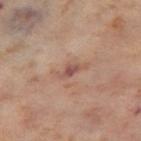Findings:
* notes · no biopsy performed (imaged during a skin exam)
* site · the right thigh
* subject · female, in their mid- to late 50s
* illumination · cross-polarized illumination
* image source · 15 mm crop, total-body photography